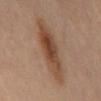Impression: Part of a total-body skin-imaging series; this lesion was reviewed on a skin check and was not flagged for biopsy. Context: A 15 mm close-up extracted from a 3D total-body photography capture. From the back. The lesion's longest dimension is about 8.5 mm. Automated tile analysis of the lesion measured an eccentricity of roughly 0.95 and a shape-asymmetry score of about 0.15 (0 = symmetric). It also reported an average lesion color of about L≈47 a*≈20 b*≈30 (CIELAB), roughly 11 lightness units darker than nearby skin, and a normalized border contrast of about 8.5. The analysis additionally found a border-irregularity rating of about 3/10, internal color variation of about 6.5 on a 0–10 scale, and radial color variation of about 2.